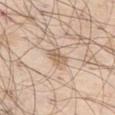<tbp_lesion>
<biopsy_status>not biopsied; imaged during a skin examination</biopsy_status>
<lesion_size>
  <long_diameter_mm_approx>3.0</long_diameter_mm_approx>
</lesion_size>
<image>
  <source>total-body photography crop</source>
  <field_of_view_mm>15</field_of_view_mm>
</image>
<patient>
  <sex>male</sex>
  <age_approx>70</age_approx>
</patient>
<lighting>white-light</lighting>
<site>left thigh</site>
</tbp_lesion>